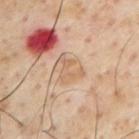Cropped from a whole-body photographic skin survey; the tile spans about 15 mm. The lesion is located on the chest. The patient is a male in their mid-50s. Automated image analysis of the tile measured roughly 7 lightness units darker than nearby skin and a normalized border contrast of about 5. The analysis additionally found border irregularity of about 5 on a 0–10 scale, a color-variation rating of about 3/10, and radial color variation of about 1. And it measured lesion-presence confidence of about 100/100.